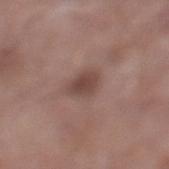An algorithmic analysis of the crop reported a footprint of about 5.5 mm² and an outline eccentricity of about 0.6 (0 = round, 1 = elongated). The analysis additionally found a classifier nevus-likeness of about 25/100 and lesion-presence confidence of about 100/100.
Approximately 3 mm at its widest.
A male subject, aged 53 to 57.
On the left lower leg.
A close-up tile cropped from a whole-body skin photograph, about 15 mm across.
Captured under white-light illumination.